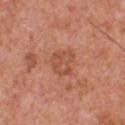Imaged during a routine full-body skin examination; the lesion was not biopsied and no histopathology is available. Automated image analysis of the tile measured a footprint of about 8 mm², an eccentricity of roughly 0.55, and a symmetry-axis asymmetry near 0.3. It also reported an average lesion color of about L≈52 a*≈27 b*≈33 (CIELAB), about 7 CIELAB-L* units darker than the surrounding skin, and a lesion-to-skin contrast of about 5.5 (normalized; higher = more distinct). The patient is a male approximately 55 years of age. Measured at roughly 3.5 mm in maximum diameter. A region of skin cropped from a whole-body photographic capture, roughly 15 mm wide. On the chest. Captured under white-light illumination.